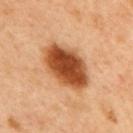Clinical impression:
Imaged during a routine full-body skin examination; the lesion was not biopsied and no histopathology is available.
Context:
About 6.5 mm across. The lesion is on the mid back. A close-up tile cropped from a whole-body skin photograph, about 15 mm across. A male patient approximately 50 years of age. Automated image analysis of the tile measured a lesion-detection confidence of about 100/100.